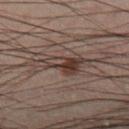lighting: cross-polarized
site: leg
image:
  source: total-body photography crop
  field_of_view_mm: 15
patient:
  sex: male
  age_approx: 40
automated_metrics:
  area_mm2_approx: 10.0
  eccentricity: 0.9
  shape_asymmetry: 0.5
  cielab_L: 29
  cielab_a: 12
  cielab_b: 17
  vs_skin_contrast_norm: 7.5